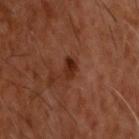acquisition — ~15 mm tile from a whole-body skin photo
diameter — ≈3 mm
subject — male, in their 60s
site — the head or neck